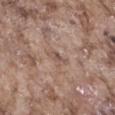<record>
  <biopsy_status>not biopsied; imaged during a skin examination</biopsy_status>
</record>